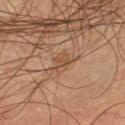notes: total-body-photography surveillance lesion; no biopsy | patient: male, approximately 55 years of age | image source: ~15 mm tile from a whole-body skin photo | diameter: about 3 mm | lighting: cross-polarized | automated lesion analysis: a lesion area of about 4 mm², an outline eccentricity of about 0.75 (0 = round, 1 = elongated), and a symmetry-axis asymmetry near 0.25; a border-irregularity index near 2.5/10 and a within-lesion color-variation index near 2.5/10; a classifier nevus-likeness of about 0/100 and a lesion-detection confidence of about 95/100 | location: the leg.